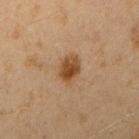Clinical impression: The lesion was photographed on a routine skin check and not biopsied; there is no pathology result. Clinical summary: The total-body-photography lesion software estimated a border-irregularity index near 1.5/10, a within-lesion color-variation index near 4.5/10, and a peripheral color-asymmetry measure near 1.5. The software also gave a nevus-likeness score of about 95/100 and lesion-presence confidence of about 100/100. This is a cross-polarized tile. About 3.5 mm across. A close-up tile cropped from a whole-body skin photograph, about 15 mm across. A female subject, about 20 years old. The lesion is on the right upper arm.